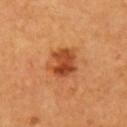{"biopsy_status": "not biopsied; imaged during a skin examination", "site": "left upper arm", "image": {"source": "total-body photography crop", "field_of_view_mm": 15}, "automated_metrics": {"area_mm2_approx": 10.0, "eccentricity": 0.4, "shape_asymmetry": 0.25, "cielab_L": 50, "cielab_a": 31, "cielab_b": 42, "vs_skin_contrast_norm": 8.5, "lesion_detection_confidence_0_100": 100}, "lesion_size": {"long_diameter_mm_approx": 3.5}, "patient": {"sex": "female", "age_approx": 60}}